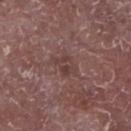Part of a total-body skin-imaging series; this lesion was reviewed on a skin check and was not flagged for biopsy.
A male patient, aged around 65.
Captured under white-light illumination.
An algorithmic analysis of the crop reported a lesion-to-skin contrast of about 6 (normalized; higher = more distinct). It also reported a peripheral color-asymmetry measure near 1.5.
A 15 mm close-up tile from a total-body photography series done for melanoma screening.
On the right lower leg.